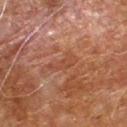  biopsy_status: not biopsied; imaged during a skin examination
  patient:
    sex: male
    age_approx: 70
  lighting: cross-polarized
  site: right forearm
  image:
    source: total-body photography crop
    field_of_view_mm: 15